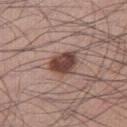• notes — no biopsy performed (imaged during a skin exam)
• site — the right thigh
• imaging modality — ~15 mm tile from a whole-body skin photo
• patient — male, aged around 35